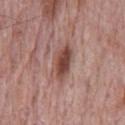Notes:
- follow-up · total-body-photography surveillance lesion; no biopsy
- patient · male, about 70 years old
- lighting · white-light
- image · ~15 mm tile from a whole-body skin photo
- automated lesion analysis · a shape eccentricity near 0.9; an average lesion color of about L≈47 a*≈22 b*≈24 (CIELAB), about 13 CIELAB-L* units darker than the surrounding skin, and a normalized border contrast of about 9.5; border irregularity of about 2.5 on a 0–10 scale, a color-variation rating of about 5/10, and a peripheral color-asymmetry measure near 2; an automated nevus-likeness rating near 95 out of 100 and a detector confidence of about 100 out of 100 that the crop contains a lesion
- location · the chest